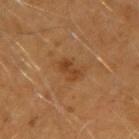Captured during whole-body skin photography for melanoma surveillance; the lesion was not biopsied. The lesion's longest dimension is about 2.5 mm. On the right upper arm. A 15 mm close-up extracted from a 3D total-body photography capture. Imaged with cross-polarized lighting. A male patient aged approximately 40.